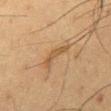The lesion was tiled from a total-body skin photograph and was not biopsied. A male patient aged around 60. Cropped from a total-body skin-imaging series; the visible field is about 15 mm. Longest diameter approximately 4 mm. Imaged with cross-polarized lighting. On the mid back.